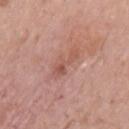Findings:
– biopsy status: no biopsy performed (imaged during a skin exam)
– patient: female, aged 63–67
– automated lesion analysis: a footprint of about 4.5 mm², an eccentricity of roughly 0.9, and two-axis asymmetry of about 0.4; a border-irregularity rating of about 6/10, internal color variation of about 2 on a 0–10 scale, and a peripheral color-asymmetry measure near 0.5
– acquisition: ~15 mm tile from a whole-body skin photo
– lighting: white-light
– lesion diameter: ~3.5 mm (longest diameter)
– body site: the left upper arm